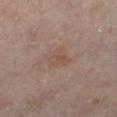Q: What are the patient's age and sex?
A: female, aged around 55
Q: What did automated image analysis measure?
A: a shape eccentricity near 0.65; a lesion color around L≈51 a*≈18 b*≈26 in CIELAB and a normalized lesion–skin contrast near 5; a nevus-likeness score of about 0/100 and a detector confidence of about 100 out of 100 that the crop contains a lesion
Q: Lesion location?
A: the left lower leg
Q: What is the imaging modality?
A: total-body-photography crop, ~15 mm field of view
Q: What is the lesion's diameter?
A: ~3 mm (longest diameter)